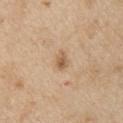Q: Is there a histopathology result?
A: catalogued during a skin exam; not biopsied
Q: How was this image acquired?
A: ~15 mm tile from a whole-body skin photo
Q: Lesion location?
A: the left upper arm
Q: Patient demographics?
A: male, aged approximately 70